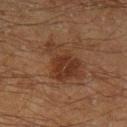tile lighting=cross-polarized | site=the left lower leg | lesion diameter=≈5.5 mm | acquisition=~15 mm tile from a whole-body skin photo | subject=male, aged approximately 60.